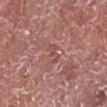workup=imaged on a skin check; not biopsied
location=the right lower leg
tile lighting=white-light
TBP lesion metrics=an area of roughly 3 mm² and an eccentricity of roughly 0.85; an average lesion color of about L≈50 a*≈24 b*≈25 (CIELAB) and a lesion–skin lightness drop of about 6
image source=total-body-photography crop, ~15 mm field of view
subject=male, aged approximately 75